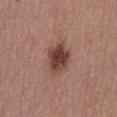<record>
  <biopsy_status>not biopsied; imaged during a skin examination</biopsy_status>
  <lighting>white-light</lighting>
  <lesion_size>
    <long_diameter_mm_approx>4.5</long_diameter_mm_approx>
  </lesion_size>
  <site>chest</site>
  <image>
    <source>total-body photography crop</source>
    <field_of_view_mm>15</field_of_view_mm>
  </image>
  <automated_metrics>
    <area_mm2_approx>11.0</area_mm2_approx>
    <eccentricity>0.7</eccentricity>
    <shape_asymmetry>0.15</shape_asymmetry>
    <border_irregularity_0_10>2.0</border_irregularity_0_10>
    <color_variation_0_10>5.0</color_variation_0_10>
  </automated_metrics>
  <patient>
    <sex>male</sex>
    <age_approx>40</age_approx>
  </patient>
</record>